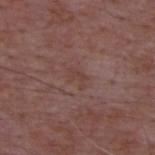Notes:
* follow-up — imaged on a skin check; not biopsied
* subject — male, aged around 50
* image source — ~15 mm crop, total-body skin-cancer survey
* size — ~2.5 mm (longest diameter)
* anatomic site — the upper back
* automated metrics — an outline eccentricity of about 0.85 (0 = round, 1 = elongated) and a shape-asymmetry score of about 0.5 (0 = symmetric); an average lesion color of about L≈41 a*≈19 b*≈22 (CIELAB), about 5 CIELAB-L* units darker than the surrounding skin, and a normalized border contrast of about 5; a border-irregularity rating of about 5.5/10, internal color variation of about 0 on a 0–10 scale, and peripheral color asymmetry of about 0; a classifier nevus-likeness of about 0/100
* lighting — white-light